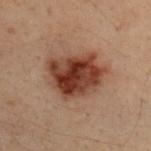The lesion was photographed on a routine skin check and not biopsied; there is no pathology result.
A close-up tile cropped from a whole-body skin photograph, about 15 mm across.
Automated image analysis of the tile measured an area of roughly 22 mm², an eccentricity of roughly 0.65, and a symmetry-axis asymmetry near 0.2. The software also gave an average lesion color of about L≈32 a*≈20 b*≈24 (CIELAB), roughly 13 lightness units darker than nearby skin, and a normalized border contrast of about 12.
A male subject, aged 28–32.
The lesion is located on the upper back.
Longest diameter approximately 6.5 mm.
This is a cross-polarized tile.